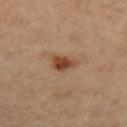Assessment: This lesion was catalogued during total-body skin photography and was not selected for biopsy. Background: A male patient, aged approximately 65. The recorded lesion diameter is about 3 mm. A close-up tile cropped from a whole-body skin photograph, about 15 mm across. This is a cross-polarized tile. The lesion is on the right thigh.